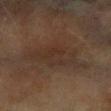The lesion was photographed on a routine skin check and not biopsied; there is no pathology result.
A female subject roughly 60 years of age.
The lesion's longest dimension is about 8 mm.
From the left forearm.
This image is a 15 mm lesion crop taken from a total-body photograph.
The tile uses cross-polarized illumination.